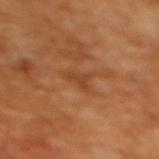Case summary:
– follow-up — total-body-photography surveillance lesion; no biopsy
– subject — female, in their mid-50s
– location — the upper back
– acquisition — ~15 mm tile from a whole-body skin photo